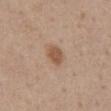No biopsy was performed on this lesion — it was imaged during a full skin examination and was not determined to be concerning. A male patient roughly 60 years of age. A close-up tile cropped from a whole-body skin photograph, about 15 mm across. Captured under white-light illumination. From the abdomen. About 2.5 mm across.